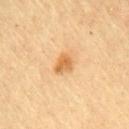biopsy status = no biopsy performed (imaged during a skin exam) | lesion diameter = about 2.5 mm | location = the front of the torso | tile lighting = cross-polarized illumination | patient = male, about 60 years old | automated lesion analysis = a border-irregularity rating of about 2.5/10, internal color variation of about 2 on a 0–10 scale, and peripheral color asymmetry of about 0.5; a classifier nevus-likeness of about 90/100 and a detector confidence of about 100 out of 100 that the crop contains a lesion | image = ~15 mm tile from a whole-body skin photo.